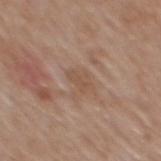Part of a total-body skin-imaging series; this lesion was reviewed on a skin check and was not flagged for biopsy.
A region of skin cropped from a whole-body photographic capture, roughly 15 mm wide.
Located on the mid back.
The total-body-photography lesion software estimated roughly 6 lightness units darker than nearby skin and a normalized border contrast of about 4.5. And it measured a color-variation rating of about 1/10 and a peripheral color-asymmetry measure near 0.5.
Imaged with white-light lighting.
The subject is a male in their 60s.
Longest diameter approximately 2.5 mm.